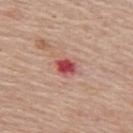| field | value |
|---|---|
| notes | total-body-photography surveillance lesion; no biopsy |
| patient | female, about 65 years old |
| diameter | about 2.5 mm |
| site | the upper back |
| TBP lesion metrics | an area of roughly 4 mm², a shape eccentricity near 0.7, and a shape-asymmetry score of about 0.25 (0 = symmetric) |
| image source | ~15 mm crop, total-body skin-cancer survey |